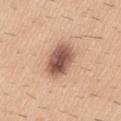Imaged during a routine full-body skin examination; the lesion was not biopsied and no histopathology is available.
Longest diameter approximately 5 mm.
A 15 mm crop from a total-body photograph taken for skin-cancer surveillance.
Captured under white-light illumination.
A male patient aged around 40.
The lesion is located on the lower back.